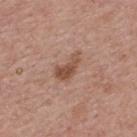Clinical impression: Part of a total-body skin-imaging series; this lesion was reviewed on a skin check and was not flagged for biopsy. Acquisition and patient details: The total-body-photography lesion software estimated an outline eccentricity of about 0.85 (0 = round, 1 = elongated) and a shape-asymmetry score of about 0.45 (0 = symmetric). And it measured a lesion color around L≈51 a*≈21 b*≈28 in CIELAB and a lesion–skin lightness drop of about 10. The software also gave border irregularity of about 4.5 on a 0–10 scale and a peripheral color-asymmetry measure near 1.5. A 15 mm close-up tile from a total-body photography series done for melanoma screening. The tile uses white-light illumination. Measured at roughly 4 mm in maximum diameter. The lesion is located on the upper back. A female subject aged 63–67.